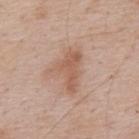Clinical impression: No biopsy was performed on this lesion — it was imaged during a full skin examination and was not determined to be concerning. Clinical summary: Located on the back. Cropped from a total-body skin-imaging series; the visible field is about 15 mm. An algorithmic analysis of the crop reported an average lesion color of about L≈58 a*≈20 b*≈29 (CIELAB), a lesion–skin lightness drop of about 9, and a lesion-to-skin contrast of about 6.5 (normalized; higher = more distinct). Imaged with white-light lighting. A male patient aged 58–62.